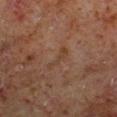Captured during whole-body skin photography for melanoma surveillance; the lesion was not biopsied.
The lesion-visualizer software estimated border irregularity of about 7 on a 0–10 scale, a color-variation rating of about 0/10, and peripheral color asymmetry of about 0.
The lesion is located on the right lower leg.
A male subject about 60 years old.
A 15 mm crop from a total-body photograph taken for skin-cancer surveillance.
Imaged with cross-polarized lighting.
Approximately 3.5 mm at its widest.